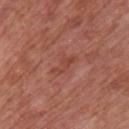Image and clinical context: Imaged with white-light lighting. Measured at roughly 3.5 mm in maximum diameter. A 15 mm crop from a total-body photograph taken for skin-cancer surveillance. The patient is a male about 70 years old. On the chest.